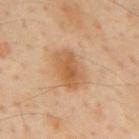biopsy_status: not biopsied; imaged during a skin examination
patient:
  sex: male
  age_approx: 55
site: mid back
image:
  source: total-body photography crop
  field_of_view_mm: 15